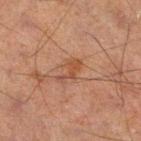The lesion was tiled from a total-body skin photograph and was not biopsied. This image is a 15 mm lesion crop taken from a total-body photograph. From the left leg. The subject is a male aged around 60. This is a cross-polarized tile. Longest diameter approximately 3.5 mm.